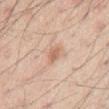Imaged during a routine full-body skin examination; the lesion was not biopsied and no histopathology is available.
The lesion is located on the mid back.
The lesion-visualizer software estimated a footprint of about 3.5 mm², an eccentricity of roughly 0.8, and a shape-asymmetry score of about 0.15 (0 = symmetric). And it measured a border-irregularity rating of about 1.5/10, internal color variation of about 2 on a 0–10 scale, and radial color variation of about 0.5. The software also gave a nevus-likeness score of about 5/100 and a lesion-detection confidence of about 100/100.
A 15 mm close-up extracted from a 3D total-body photography capture.
Imaged with white-light lighting.
A male patient, approximately 45 years of age.